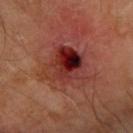Assessment: The lesion was photographed on a routine skin check and not biopsied; there is no pathology result. Acquisition and patient details: Measured at roughly 6.5 mm in maximum diameter. A roughly 15 mm field-of-view crop from a total-body skin photograph. A male patient, aged around 70. Captured under cross-polarized illumination. The lesion is located on the arm.